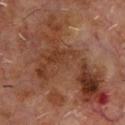follow-up — catalogued during a skin exam; not biopsied
image-analysis metrics — a lesion color around L≈37 a*≈21 b*≈27 in CIELAB, a lesion–skin lightness drop of about 8, and a normalized lesion–skin contrast near 7.5; border irregularity of about 8.5 on a 0–10 scale and a peripheral color-asymmetry measure near 3
lighting — cross-polarized illumination
patient — male, aged around 60
anatomic site — the chest
diameter — about 14.5 mm
image — ~15 mm tile from a whole-body skin photo